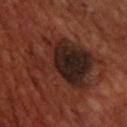Clinical impression: Captured during whole-body skin photography for melanoma surveillance; the lesion was not biopsied. Image and clinical context: Located on the back. Longest diameter approximately 10 mm. A male subject, in their 70s. A 15 mm crop from a total-body photograph taken for skin-cancer surveillance. Captured under cross-polarized illumination. Automated image analysis of the tile measured a footprint of about 35 mm² and a symmetry-axis asymmetry near 0.5. And it measured about 9 CIELAB-L* units darker than the surrounding skin. It also reported a border-irregularity index near 8.5/10, a within-lesion color-variation index near 8.5/10, and peripheral color asymmetry of about 2.5.